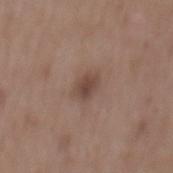patient:
  sex: male
  age_approx: 50
site: mid back
image:
  source: total-body photography crop
  field_of_view_mm: 15